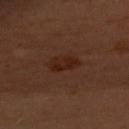Captured during whole-body skin photography for melanoma surveillance; the lesion was not biopsied. The lesion's longest dimension is about 3.5 mm. Captured under cross-polarized illumination. A female subject, aged approximately 50. A lesion tile, about 15 mm wide, cut from a 3D total-body photograph. The lesion is on the chest.